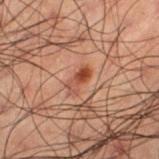Case summary:
- image-analysis metrics: a lesion color around L≈35 a*≈21 b*≈26 in CIELAB, about 9 CIELAB-L* units darker than the surrounding skin, and a lesion-to-skin contrast of about 8 (normalized; higher = more distinct); border irregularity of about 3.5 on a 0–10 scale and a peripheral color-asymmetry measure near 0
- lighting: cross-polarized illumination
- image source: ~15 mm crop, total-body skin-cancer survey
- anatomic site: the left thigh
- subject: male, aged 48 to 52